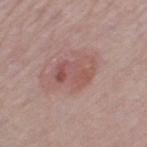No biopsy was performed on this lesion — it was imaged during a full skin examination and was not determined to be concerning.
From the left thigh.
Captured under white-light illumination.
The total-body-photography lesion software estimated a footprint of about 9 mm².
A 15 mm close-up extracted from a 3D total-body photography capture.
About 4.5 mm across.
A female patient, aged around 65.